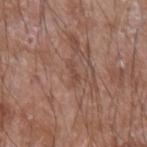notes: total-body-photography surveillance lesion; no biopsy
subject: male, approximately 60 years of age
imaging modality: 15 mm crop, total-body photography
anatomic site: the left forearm